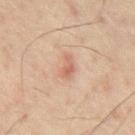workup = imaged on a skin check; not biopsied
size = ≈3 mm
image source = 15 mm crop, total-body photography
location = the abdomen
lighting = cross-polarized
patient = male, aged 58–62
TBP lesion metrics = an area of roughly 4 mm², a shape eccentricity near 0.85, and a symmetry-axis asymmetry near 0.35; a mean CIELAB color near L≈55 a*≈20 b*≈27 and a normalized lesion–skin contrast near 5.5; a within-lesion color-variation index near 1.5/10; a classifier nevus-likeness of about 5/100 and lesion-presence confidence of about 100/100